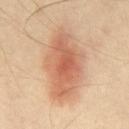<case>
  <biopsy_status>not biopsied; imaged during a skin examination</biopsy_status>
  <lighting>cross-polarized</lighting>
  <image>
    <source>total-body photography crop</source>
    <field_of_view_mm>15</field_of_view_mm>
  </image>
  <automated_metrics>
    <area_mm2_approx>30.0</area_mm2_approx>
    <eccentricity>0.85</eccentricity>
    <shape_asymmetry>0.15</shape_asymmetry>
    <cielab_L>62</cielab_L>
    <cielab_a>23</cielab_a>
    <cielab_b>33</cielab_b>
    <vs_skin_darker_L>12.0</vs_skin_darker_L>
    <vs_skin_contrast_norm>7.5</vs_skin_contrast_norm>
    <border_irregularity_0_10>2.0</border_irregularity_0_10>
    <color_variation_0_10>6.0</color_variation_0_10>
    <peripheral_color_asymmetry>1.5</peripheral_color_asymmetry>
  </automated_metrics>
  <site>abdomen</site>
  <patient>
    <sex>male</sex>
    <age_approx>65</age_approx>
  </patient>
  <lesion_size>
    <long_diameter_mm_approx>8.5</long_diameter_mm_approx>
  </lesion_size>
</case>